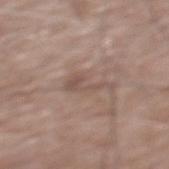Case summary:
– biopsy status: no biopsy performed (imaged during a skin exam)
– image source: ~15 mm tile from a whole-body skin photo
– size: about 4.5 mm
– image-analysis metrics: a lesion area of about 4.5 mm², an outline eccentricity of about 0.95 (0 = round, 1 = elongated), and a symmetry-axis asymmetry near 0.65
– body site: the mid back
– illumination: white-light
– subject: male, aged 58–62